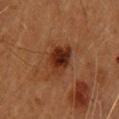Recorded during total-body skin imaging; not selected for excision or biopsy. A female patient aged approximately 60. An algorithmic analysis of the crop reported a lesion color around L≈27 a*≈22 b*≈28 in CIELAB, a lesion–skin lightness drop of about 11, and a lesion-to-skin contrast of about 10.5 (normalized; higher = more distinct). A roughly 15 mm field-of-view crop from a total-body skin photograph. Imaged with cross-polarized lighting. From the upper back.